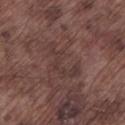Q: How was this image acquired?
A: ~15 mm crop, total-body skin-cancer survey
Q: Lesion location?
A: the left lower leg
Q: Who is the patient?
A: male, roughly 75 years of age
Q: Lesion size?
A: about 5.5 mm